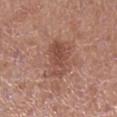A 15 mm close-up extracted from a 3D total-body photography capture. Longest diameter approximately 4.5 mm. The lesion is located on the right lower leg. The subject is a female about 40 years old. Captured under white-light illumination.